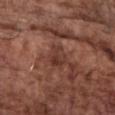The lesion was tiled from a total-body skin photograph and was not biopsied.